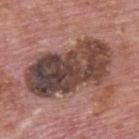Assessment: Captured during whole-body skin photography for melanoma surveillance; the lesion was not biopsied. Acquisition and patient details: A male patient aged approximately 75. This is a white-light tile. The lesion is on the back. Approximately 10 mm at its widest. The lesion-visualizer software estimated an area of roughly 42 mm² and an outline eccentricity of about 0.9 (0 = round, 1 = elongated). The analysis additionally found an average lesion color of about L≈42 a*≈18 b*≈22 (CIELAB) and a lesion–skin lightness drop of about 16. It also reported a border-irregularity rating of about 3/10, internal color variation of about 10 on a 0–10 scale, and a peripheral color-asymmetry measure near 3. A close-up tile cropped from a whole-body skin photograph, about 15 mm across.